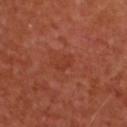Assessment: Part of a total-body skin-imaging series; this lesion was reviewed on a skin check and was not flagged for biopsy. Acquisition and patient details: A 15 mm close-up extracted from a 3D total-body photography capture. The lesion is located on the upper back. Automated image analysis of the tile measured an automated nevus-likeness rating near 0 out of 100 and lesion-presence confidence of about 100/100. A male patient aged around 50. Imaged with cross-polarized lighting.